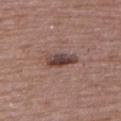Q: Was a biopsy performed?
A: total-body-photography surveillance lesion; no biopsy
Q: What is the imaging modality?
A: ~15 mm tile from a whole-body skin photo
Q: How was the tile lit?
A: white-light illumination
Q: What is the anatomic site?
A: the upper back
Q: Who is the patient?
A: female, about 65 years old